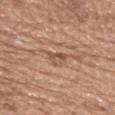  biopsy_status: not biopsied; imaged during a skin examination
  lighting: white-light
  site: upper back
  image:
    source: total-body photography crop
    field_of_view_mm: 15
  patient:
    sex: female
    age_approx: 65